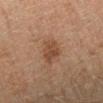<case>
<biopsy_status>not biopsied; imaged during a skin examination</biopsy_status>
<lesion_size>
  <long_diameter_mm_approx>3.0</long_diameter_mm_approx>
</lesion_size>
<patient>
  <sex>male</sex>
  <age_approx>65</age_approx>
</patient>
<image>
  <source>total-body photography crop</source>
  <field_of_view_mm>15</field_of_view_mm>
</image>
<site>left lower leg</site>
<automated_metrics>
  <border_irregularity_0_10>2.5</border_irregularity_0_10>
  <color_variation_0_10>2.0</color_variation_0_10>
  <peripheral_color_asymmetry>0.5</peripheral_color_asymmetry>
  <nevus_likeness_0_100>25</nevus_likeness_0_100>
  <lesion_detection_confidence_0_100>100</lesion_detection_confidence_0_100>
</automated_metrics>
<lighting>cross-polarized</lighting>
</case>